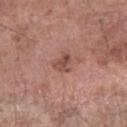Q: Was this lesion biopsied?
A: no biopsy performed (imaged during a skin exam)
Q: What is the imaging modality?
A: total-body-photography crop, ~15 mm field of view
Q: What are the patient's age and sex?
A: male, in their 60s
Q: Where on the body is the lesion?
A: the right forearm
Q: What did automated image analysis measure?
A: a footprint of about 4 mm², a shape eccentricity near 0.65, and two-axis asymmetry of about 0.4; a within-lesion color-variation index near 4/10 and peripheral color asymmetry of about 1.5